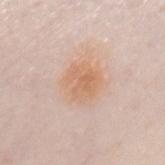follow-up — catalogued during a skin exam; not biopsied
illumination — white-light
image-analysis metrics — a footprint of about 11 mm², a shape eccentricity near 0.35, and a shape-asymmetry score of about 0.15 (0 = symmetric); a mean CIELAB color near L≈66 a*≈20 b*≈33 and about 7 CIELAB-L* units darker than the surrounding skin; a nevus-likeness score of about 100/100 and a lesion-detection confidence of about 100/100
size — about 4 mm
anatomic site — the chest
imaging modality — total-body-photography crop, ~15 mm field of view
patient — female, aged 68 to 72